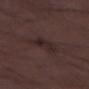Q: Is there a histopathology result?
A: catalogued during a skin exam; not biopsied
Q: How was this image acquired?
A: 15 mm crop, total-body photography
Q: Patient demographics?
A: male, about 50 years old
Q: Lesion location?
A: the leg
Q: Illumination type?
A: white-light illumination
Q: What is the lesion's diameter?
A: ≈5 mm
Q: Automated lesion metrics?
A: an area of roughly 7 mm² and an outline eccentricity of about 0.9 (0 = round, 1 = elongated); a mean CIELAB color near L≈24 a*≈15 b*≈15 and a normalized lesion–skin contrast near 6.5; a border-irregularity rating of about 4.5/10 and a peripheral color-asymmetry measure near 1; an automated nevus-likeness rating near 10 out of 100 and lesion-presence confidence of about 55/100